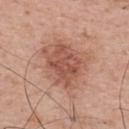The lesion was tiled from a total-body skin photograph and was not biopsied. The patient is a male in their mid- to late 60s. Automated tile analysis of the lesion measured an average lesion color of about L≈55 a*≈23 b*≈29 (CIELAB), about 10 CIELAB-L* units darker than the surrounding skin, and a normalized lesion–skin contrast near 7. The analysis additionally found border irregularity of about 3 on a 0–10 scale, a within-lesion color-variation index near 4.5/10, and radial color variation of about 1.5. It also reported lesion-presence confidence of about 100/100. On the upper back. The tile uses white-light illumination. A region of skin cropped from a whole-body photographic capture, roughly 15 mm wide. The recorded lesion diameter is about 6 mm.